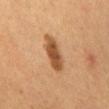<record>
  <biopsy_status>not biopsied; imaged during a skin examination</biopsy_status>
  <site>abdomen</site>
  <image>
    <source>total-body photography crop</source>
    <field_of_view_mm>15</field_of_view_mm>
  </image>
  <lesion_size>
    <long_diameter_mm_approx>5.0</long_diameter_mm_approx>
  </lesion_size>
  <patient>
    <sex>male</sex>
    <age_approx>55</age_approx>
  </patient>
</record>